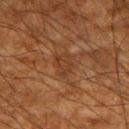A male patient aged 63–67. From the right upper arm. A roughly 15 mm field-of-view crop from a total-body skin photograph.